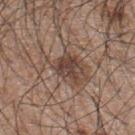The lesion-visualizer software estimated a lesion area of about 5 mm² and a symmetry-axis asymmetry near 0.3.
About 2.5 mm across.
The tile uses white-light illumination.
This image is a 15 mm lesion crop taken from a total-body photograph.
The patient is a male aged 43–47.
The lesion is on the upper back.